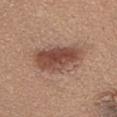<lesion>
  <biopsy_status>not biopsied; imaged during a skin examination</biopsy_status>
  <lesion_size>
    <long_diameter_mm_approx>6.0</long_diameter_mm_approx>
  </lesion_size>
  <patient>
    <sex>male</sex>
    <age_approx>60</age_approx>
  </patient>
  <automated_metrics>
    <border_irregularity_0_10>2.5</border_irregularity_0_10>
    <peripheral_color_asymmetry>2.0</peripheral_color_asymmetry>
    <nevus_likeness_0_100>90</nevus_likeness_0_100>
    <lesion_detection_confidence_0_100>100</lesion_detection_confidence_0_100>
  </automated_metrics>
  <lighting>white-light</lighting>
  <image>
    <source>total-body photography crop</source>
    <field_of_view_mm>15</field_of_view_mm>
  </image>
  <site>upper back</site>
</lesion>